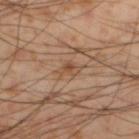workup: total-body-photography surveillance lesion; no biopsy
image: total-body-photography crop, ~15 mm field of view
diameter: ≈2.5 mm
site: the leg
tile lighting: cross-polarized illumination
patient: male, aged around 55